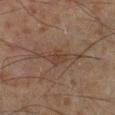notes = no biopsy performed (imaged during a skin exam)
lighting = cross-polarized
site = the left lower leg
subject = male, roughly 65 years of age
lesion diameter = about 7 mm
image = ~15 mm tile from a whole-body skin photo